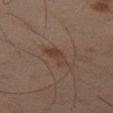| key | value |
|---|---|
| lesion size | about 3.5 mm |
| imaging modality | ~15 mm tile from a whole-body skin photo |
| patient | male, aged 43 to 47 |
| TBP lesion metrics | a lesion area of about 5 mm² and a shape-asymmetry score of about 0.4 (0 = symmetric); border irregularity of about 4 on a 0–10 scale, a within-lesion color-variation index near 3.5/10, and peripheral color asymmetry of about 1; an automated nevus-likeness rating near 10 out of 100 and a detector confidence of about 100 out of 100 that the crop contains a lesion |
| site | the left thigh |
| illumination | cross-polarized illumination |